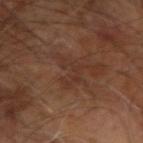- workup — catalogued during a skin exam; not biopsied
- TBP lesion metrics — a footprint of about 5.5 mm², an eccentricity of roughly 0.8, and a symmetry-axis asymmetry near 0.65; an average lesion color of about L≈31 a*≈18 b*≈24 (CIELAB), about 5 CIELAB-L* units darker than the surrounding skin, and a normalized lesion–skin contrast near 5; border irregularity of about 10 on a 0–10 scale, a color-variation rating of about 0.5/10, and peripheral color asymmetry of about 0
- patient — male, in their 60s
- size — ≈4 mm
- body site — the right arm
- lighting — cross-polarized
- image — 15 mm crop, total-body photography